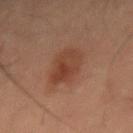| field | value |
|---|---|
| follow-up | imaged on a skin check; not biopsied |
| illumination | cross-polarized illumination |
| lesion diameter | ≈5 mm |
| image-analysis metrics | a lesion area of about 12 mm², a shape eccentricity near 0.85, and a shape-asymmetry score of about 0.15 (0 = symmetric); an average lesion color of about L≈35 a*≈19 b*≈26 (CIELAB), about 7 CIELAB-L* units darker than the surrounding skin, and a lesion-to-skin contrast of about 7 (normalized; higher = more distinct); a nevus-likeness score of about 95/100 and lesion-presence confidence of about 100/100 |
| acquisition | ~15 mm tile from a whole-body skin photo |
| subject | male, aged 53 to 57 |
| body site | the abdomen |